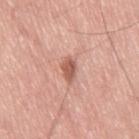Clinical impression: This lesion was catalogued during total-body skin photography and was not selected for biopsy. Acquisition and patient details: On the right thigh. This is a white-light tile. A male subject aged 68–72. A 15 mm close-up extracted from a 3D total-body photography capture. The lesion's longest dimension is about 3 mm.